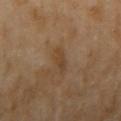Findings:
- follow-up: no biopsy performed (imaged during a skin exam)